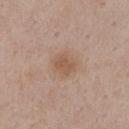| key | value |
|---|---|
| follow-up | catalogued during a skin exam; not biopsied |
| illumination | white-light illumination |
| image source | 15 mm crop, total-body photography |
| patient | female, roughly 55 years of age |
| anatomic site | the chest |